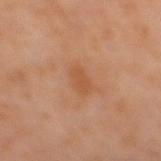{"biopsy_status": "not biopsied; imaged during a skin examination", "image": {"source": "total-body photography crop", "field_of_view_mm": 15}, "lesion_size": {"long_diameter_mm_approx": 3.0}, "patient": {"sex": "male", "age_approx": 60}, "lighting": "cross-polarized", "site": "mid back"}